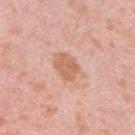Approximately 4 mm at its widest. On the left upper arm. Cropped from a total-body skin-imaging series; the visible field is about 15 mm. The patient is a female approximately 40 years of age. This is a white-light tile.